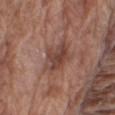Part of a total-body skin-imaging series; this lesion was reviewed on a skin check and was not flagged for biopsy. An algorithmic analysis of the crop reported a lesion area of about 8.5 mm², an outline eccentricity of about 0.5 (0 = round, 1 = elongated), and two-axis asymmetry of about 0.35. The tile uses white-light illumination. A male subject aged approximately 75. Located on the right upper arm. Longest diameter approximately 3.5 mm. This image is a 15 mm lesion crop taken from a total-body photograph.